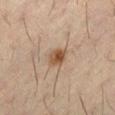This lesion was catalogued during total-body skin photography and was not selected for biopsy. The subject is a male in their 50s. This is a cross-polarized tile. About 2.5 mm across. From the left thigh. Cropped from a total-body skin-imaging series; the visible field is about 15 mm.